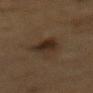Clinical impression:
No biopsy was performed on this lesion — it was imaged during a full skin examination and was not determined to be concerning.
Image and clinical context:
About 5 mm across. Cropped from a whole-body photographic skin survey; the tile spans about 15 mm. The lesion is located on the mid back. The subject is a male aged around 85 or older. Imaged with cross-polarized lighting. Automated image analysis of the tile measured border irregularity of about 3.5 on a 0–10 scale, internal color variation of about 4 on a 0–10 scale, and radial color variation of about 1.